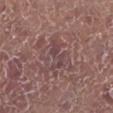<record>
  <biopsy_status>not biopsied; imaged during a skin examination</biopsy_status>
  <patient>
    <sex>male</sex>
    <age_approx>80</age_approx>
  </patient>
  <lighting>white-light</lighting>
  <image>
    <source>total-body photography crop</source>
    <field_of_view_mm>15</field_of_view_mm>
  </image>
  <site>left lower leg</site>
  <lesion_size>
    <long_diameter_mm_approx>4.5</long_diameter_mm_approx>
  </lesion_size>
</record>